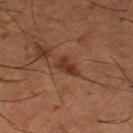follow-up=no biopsy performed (imaged during a skin exam)
location=the left thigh
patient=male, aged approximately 65
imaging modality=15 mm crop, total-body photography
lesion size=~3 mm (longest diameter)